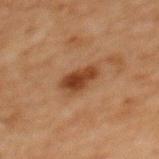• workup — catalogued during a skin exam; not biopsied
• tile lighting — cross-polarized
• size — about 4 mm
• patient — female, in their 60s
• anatomic site — the upper back
• image — total-body-photography crop, ~15 mm field of view